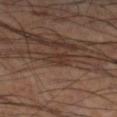No biopsy was performed on this lesion — it was imaged during a full skin examination and was not determined to be concerning.
A close-up tile cropped from a whole-body skin photograph, about 15 mm across.
The subject is a male approximately 60 years of age.
On the right lower leg.